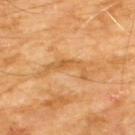Notes:
– follow-up: total-body-photography surveillance lesion; no biopsy
– lighting: cross-polarized
– image: ~15 mm crop, total-body skin-cancer survey
– lesion size: ~5.5 mm (longest diameter)
– patient: male, aged around 60
– site: the chest
– automated lesion analysis: an area of roughly 6.5 mm², an eccentricity of roughly 0.9, and two-axis asymmetry of about 0.75; a lesion color around L≈58 a*≈22 b*≈43 in CIELAB and a normalized lesion–skin contrast near 5.5; a nevus-likeness score of about 0/100 and a lesion-detection confidence of about 80/100